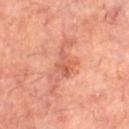The lesion was photographed on a routine skin check and not biopsied; there is no pathology result.
A 15 mm close-up extracted from a 3D total-body photography capture.
An algorithmic analysis of the crop reported a lesion area of about 5 mm² and a shape-asymmetry score of about 0.35 (0 = symmetric). And it measured a lesion color around L≈55 a*≈28 b*≈33 in CIELAB, a lesion–skin lightness drop of about 9, and a normalized border contrast of about 6. And it measured an automated nevus-likeness rating near 0 out of 100 and a detector confidence of about 100 out of 100 that the crop contains a lesion.
Approximately 3.5 mm at its widest.
This is a cross-polarized tile.
The lesion is located on the right thigh.
A male subject aged 58 to 62.